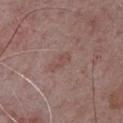Impression:
Part of a total-body skin-imaging series; this lesion was reviewed on a skin check and was not flagged for biopsy.
Acquisition and patient details:
This image is a 15 mm lesion crop taken from a total-body photograph. The lesion is located on the chest. A male patient, in their mid-60s.